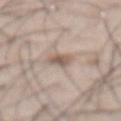This lesion was catalogued during total-body skin photography and was not selected for biopsy.
The lesion is located on the front of the torso.
The total-body-photography lesion software estimated a footprint of about 4 mm², an eccentricity of roughly 0.8, and two-axis asymmetry of about 0.25. The analysis additionally found an average lesion color of about L≈57 a*≈15 b*≈24 (CIELAB) and a lesion–skin lightness drop of about 11. The analysis additionally found a within-lesion color-variation index near 3.5/10 and a peripheral color-asymmetry measure near 1.
Approximately 3 mm at its widest.
The subject is a male aged 58–62.
A region of skin cropped from a whole-body photographic capture, roughly 15 mm wide.